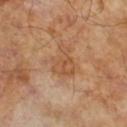Q: Was a biopsy performed?
A: total-body-photography surveillance lesion; no biopsy
Q: How was this image acquired?
A: ~15 mm tile from a whole-body skin photo
Q: Patient demographics?
A: male, approximately 65 years of age
Q: Illumination type?
A: cross-polarized